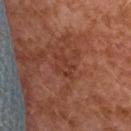Assessment:
Recorded during total-body skin imaging; not selected for excision or biopsy.
Acquisition and patient details:
Captured under cross-polarized illumination. A lesion tile, about 15 mm wide, cut from a 3D total-body photograph. The patient is a female roughly 60 years of age. On the upper back. An algorithmic analysis of the crop reported a lesion area of about 2.5 mm², an eccentricity of roughly 0.95, and a shape-asymmetry score of about 0.7 (0 = symmetric). And it measured a lesion color around L≈31 a*≈23 b*≈25 in CIELAB, a lesion–skin lightness drop of about 5, and a normalized lesion–skin contrast near 5. The software also gave peripheral color asymmetry of about 0. The software also gave a nevus-likeness score of about 0/100 and a detector confidence of about 100 out of 100 that the crop contains a lesion. About 3 mm across.